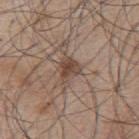patient = male, aged 53 to 57 | image source = ~15 mm tile from a whole-body skin photo | tile lighting = white-light illumination | lesion diameter = ≈3.5 mm | body site = the upper back.